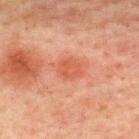| field | value |
|---|---|
| notes | total-body-photography surveillance lesion; no biopsy |
| body site | the upper back |
| patient | male, approximately 60 years of age |
| image | 15 mm crop, total-body photography |
| lighting | cross-polarized |
| image-analysis metrics | an eccentricity of roughly 0.65 and two-axis asymmetry of about 0.25; a mean CIELAB color near L≈47 a*≈28 b*≈31 and roughly 7 lightness units darker than nearby skin; a lesion-detection confidence of about 100/100 |